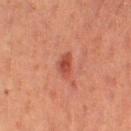| key | value |
|---|---|
| notes | catalogued during a skin exam; not biopsied |
| anatomic site | the leg |
| patient | female, aged 18–22 |
| imaging modality | ~15 mm tile from a whole-body skin photo |